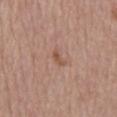{
  "biopsy_status": "not biopsied; imaged during a skin examination",
  "site": "back",
  "lesion_size": {
    "long_diameter_mm_approx": 2.5
  },
  "patient": {
    "sex": "male",
    "age_approx": 80
  },
  "image": {
    "source": "total-body photography crop",
    "field_of_view_mm": 15
  },
  "automated_metrics": {
    "eccentricity": 0.9,
    "shape_asymmetry": 0.5
  },
  "lighting": "white-light"
}